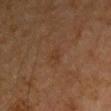site — the right upper arm | tile lighting — cross-polarized illumination | TBP lesion metrics — an area of roughly 3 mm², an eccentricity of roughly 0.75, and a symmetry-axis asymmetry near 0.4; an average lesion color of about L≈33 a*≈17 b*≈28 (CIELAB) and a lesion-to-skin contrast of about 4.5 (normalized; higher = more distinct); internal color variation of about 1.5 on a 0–10 scale and peripheral color asymmetry of about 0.5 | lesion size — about 2.5 mm | patient — male, aged approximately 60 | imaging modality — ~15 mm crop, total-body skin-cancer survey.